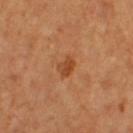Assessment: Captured during whole-body skin photography for melanoma surveillance; the lesion was not biopsied. Clinical summary: The recorded lesion diameter is about 2.5 mm. The lesion is on the right thigh. Captured under cross-polarized illumination. An algorithmic analysis of the crop reported a lesion color around L≈48 a*≈28 b*≈40 in CIELAB, roughly 9 lightness units darker than nearby skin, and a lesion-to-skin contrast of about 7.5 (normalized; higher = more distinct). And it measured a within-lesion color-variation index near 2.5/10 and peripheral color asymmetry of about 1. The analysis additionally found a classifier nevus-likeness of about 60/100. A 15 mm crop from a total-body photograph taken for skin-cancer surveillance. A female patient, in their mid- to late 50s.